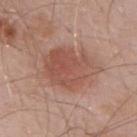Part of a total-body skin-imaging series; this lesion was reviewed on a skin check and was not flagged for biopsy.
A male patient approximately 55 years of age.
Captured under white-light illumination.
The lesion is located on the mid back.
The recorded lesion diameter is about 6.5 mm.
A 15 mm close-up tile from a total-body photography series done for melanoma screening.